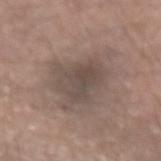Q: Is there a histopathology result?
A: total-body-photography surveillance lesion; no biopsy
Q: Lesion size?
A: about 4.5 mm
Q: Where on the body is the lesion?
A: the left forearm
Q: Illumination type?
A: white-light
Q: What kind of image is this?
A: 15 mm crop, total-body photography
Q: Who is the patient?
A: male, aged around 55
Q: What did automated image analysis measure?
A: an average lesion color of about L≈47 a*≈12 b*≈20 (CIELAB), about 8 CIELAB-L* units darker than the surrounding skin, and a normalized border contrast of about 6.5